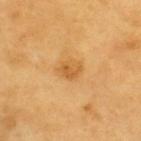Q: Was this lesion biopsied?
A: no biopsy performed (imaged during a skin exam)
Q: How was this image acquired?
A: 15 mm crop, total-body photography
Q: What lighting was used for the tile?
A: cross-polarized illumination
Q: What are the patient's age and sex?
A: male, aged 58 to 62
Q: Automated lesion metrics?
A: an area of roughly 4 mm², an outline eccentricity of about 0.65 (0 = round, 1 = elongated), and two-axis asymmetry of about 0.35; a lesion color around L≈58 a*≈22 b*≈47 in CIELAB, a lesion–skin lightness drop of about 9, and a lesion-to-skin contrast of about 6.5 (normalized; higher = more distinct); an automated nevus-likeness rating near 35 out of 100 and a lesion-detection confidence of about 100/100
Q: How large is the lesion?
A: ~2.5 mm (longest diameter)
Q: Where on the body is the lesion?
A: the upper back